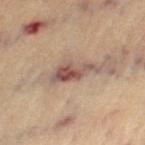{
  "biopsy_status": "not biopsied; imaged during a skin examination",
  "patient": {
    "sex": "female",
    "age_approx": 65
  },
  "image": {
    "source": "total-body photography crop",
    "field_of_view_mm": 15
  },
  "site": "left thigh",
  "lesion_size": {
    "long_diameter_mm_approx": 5.0
  },
  "automated_metrics": {
    "color_variation_0_10": 4.0,
    "peripheral_color_asymmetry": 1.0,
    "nevus_likeness_0_100": 25,
    "lesion_detection_confidence_0_100": 95
  }
}